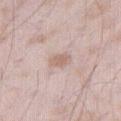<lesion>
<biopsy_status>not biopsied; imaged during a skin examination</biopsy_status>
<automated_metrics>
  <cielab_L>63</cielab_L>
  <cielab_a>17</cielab_a>
  <cielab_b>24</cielab_b>
  <vs_skin_darker_L>9.0</vs_skin_darker_L>
  <vs_skin_contrast_norm>6.0</vs_skin_contrast_norm>
  <color_variation_0_10>2.0</color_variation_0_10>
</automated_metrics>
<image>
  <source>total-body photography crop</source>
  <field_of_view_mm>15</field_of_view_mm>
</image>
<site>right thigh</site>
<patient>
  <sex>male</sex>
  <age_approx>45</age_approx>
</patient>
</lesion>